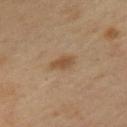<lesion>
  <biopsy_status>not biopsied; imaged during a skin examination</biopsy_status>
  <lesion_size>
    <long_diameter_mm_approx>2.5</long_diameter_mm_approx>
  </lesion_size>
  <image>
    <source>total-body photography crop</source>
    <field_of_view_mm>15</field_of_view_mm>
  </image>
  <site>chest</site>
  <automated_metrics>
    <eccentricity>0.75</eccentricity>
    <shape_asymmetry>0.25</shape_asymmetry>
    <nevus_likeness_0_100>70</nevus_likeness_0_100>
    <lesion_detection_confidence_0_100>100</lesion_detection_confidence_0_100>
  </automated_metrics>
  <patient>
    <sex>male</sex>
    <age_approx>55</age_approx>
  </patient>
</lesion>